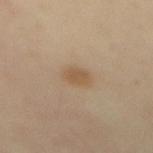biopsy_status: not biopsied; imaged during a skin examination
image:
  source: total-body photography crop
  field_of_view_mm: 15
site: back
patient:
  sex: female
  age_approx: 40